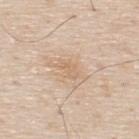Assessment:
The lesion was tiled from a total-body skin photograph and was not biopsied.
Context:
A close-up tile cropped from a whole-body skin photograph, about 15 mm across. About 2.5 mm across. Located on the upper back. The subject is a male aged approximately 80.